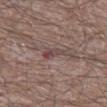Q: Was a biopsy performed?
A: imaged on a skin check; not biopsied
Q: What is the lesion's diameter?
A: ~3 mm (longest diameter)
Q: What are the patient's age and sex?
A: male, about 65 years old
Q: What did automated image analysis measure?
A: border irregularity of about 4 on a 0–10 scale, internal color variation of about 4.5 on a 0–10 scale, and a peripheral color-asymmetry measure near 1
Q: Lesion location?
A: the left thigh
Q: What kind of image is this?
A: ~15 mm crop, total-body skin-cancer survey
Q: What lighting was used for the tile?
A: white-light illumination